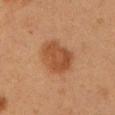Recorded during total-body skin imaging; not selected for excision or biopsy.
This is a cross-polarized tile.
A roughly 15 mm field-of-view crop from a total-body skin photograph.
Approximately 4.5 mm at its widest.
The subject is a female about 40 years old.
The total-body-photography lesion software estimated a footprint of about 14 mm², a shape eccentricity near 0.65, and two-axis asymmetry of about 0.2. It also reported about 9 CIELAB-L* units darker than the surrounding skin and a normalized lesion–skin contrast near 7.5. The analysis additionally found a border-irregularity index near 1.5/10.
From the left upper arm.